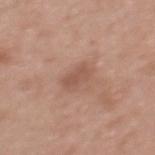Impression:
The lesion was tiled from a total-body skin photograph and was not biopsied.
Clinical summary:
The recorded lesion diameter is about 2.5 mm. The lesion-visualizer software estimated border irregularity of about 1.5 on a 0–10 scale, internal color variation of about 1.5 on a 0–10 scale, and radial color variation of about 0.5. And it measured a nevus-likeness score of about 10/100. Captured under white-light illumination. A female subject, aged approximately 35. A 15 mm crop from a total-body photograph taken for skin-cancer surveillance. From the upper back.